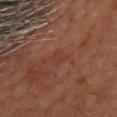The lesion was tiled from a total-body skin photograph and was not biopsied.
A 15 mm crop from a total-body photograph taken for skin-cancer surveillance.
The subject is a female approximately 60 years of age.
Approximately 2 mm at its widest.
The lesion is located on the head or neck.
Imaged with cross-polarized lighting.